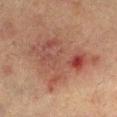Clinical impression: No biopsy was performed on this lesion — it was imaged during a full skin examination and was not determined to be concerning. Image and clinical context: Located on the leg. A region of skin cropped from a whole-body photographic capture, roughly 15 mm wide. A female subject, in their mid-50s. Measured at roughly 8.5 mm in maximum diameter. Imaged with cross-polarized lighting.